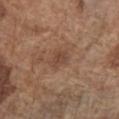Recorded during total-body skin imaging; not selected for excision or biopsy.
The tile uses white-light illumination.
Measured at roughly 2.5 mm in maximum diameter.
A 15 mm crop from a total-body photograph taken for skin-cancer surveillance.
Located on the front of the torso.
The subject is a male aged approximately 75.